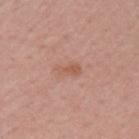Clinical impression: Part of a total-body skin-imaging series; this lesion was reviewed on a skin check and was not flagged for biopsy. Background: Automated tile analysis of the lesion measured a lesion area of about 3.5 mm² and an outline eccentricity of about 0.9 (0 = round, 1 = elongated). The analysis additionally found an average lesion color of about L≈56 a*≈23 b*≈29 (CIELAB) and about 7 CIELAB-L* units darker than the surrounding skin. The recorded lesion diameter is about 2.5 mm. From the left upper arm. This is a white-light tile. The subject is a female aged 53 to 57. Cropped from a whole-body photographic skin survey; the tile spans about 15 mm.